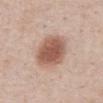Findings:
- workup · imaged on a skin check; not biopsied
- acquisition · ~15 mm tile from a whole-body skin photo
- illumination · white-light
- image-analysis metrics · lesion-presence confidence of about 100/100
- body site · the abdomen
- subject · male, aged 58–62